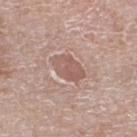{"biopsy_status": "not biopsied; imaged during a skin examination", "automated_metrics": {"eccentricity": 0.8, "border_irregularity_0_10": 2.0, "color_variation_0_10": 3.0, "peripheral_color_asymmetry": 1.0, "nevus_likeness_0_100": 0, "lesion_detection_confidence_0_100": 90}, "patient": {"sex": "male", "age_approx": 80}, "lesion_size": {"long_diameter_mm_approx": 4.0}, "image": {"source": "total-body photography crop", "field_of_view_mm": 15}, "site": "leg"}